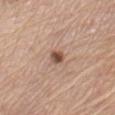The tile uses white-light illumination. The patient is a female aged 63 to 67. The lesion is on the left upper arm. Longest diameter approximately 2 mm. A 15 mm crop from a total-body photograph taken for skin-cancer surveillance. An algorithmic analysis of the crop reported border irregularity of about 2.5 on a 0–10 scale and radial color variation of about 2. The software also gave an automated nevus-likeness rating near 95 out of 100 and lesion-presence confidence of about 100/100.